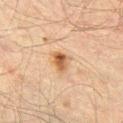Captured during whole-body skin photography for melanoma surveillance; the lesion was not biopsied.
The total-body-photography lesion software estimated a border-irregularity index near 3/10, a within-lesion color-variation index near 6/10, and a peripheral color-asymmetry measure near 2.
The lesion is on the left thigh.
The subject is a male aged around 60.
This image is a 15 mm lesion crop taken from a total-body photograph.